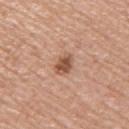{
  "biopsy_status": "not biopsied; imaged during a skin examination",
  "lighting": "white-light",
  "automated_metrics": {
    "nevus_likeness_0_100": 85,
    "lesion_detection_confidence_0_100": 100
  },
  "site": "upper back",
  "patient": {
    "sex": "male",
    "age_approx": 65
  },
  "image": {
    "source": "total-body photography crop",
    "field_of_view_mm": 15
  }
}